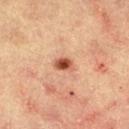Assessment: Imaged during a routine full-body skin examination; the lesion was not biopsied and no histopathology is available. Background: The patient is a male aged around 65. The lesion is on the right thigh. The lesion's longest dimension is about 2.5 mm. Captured under cross-polarized illumination. A 15 mm close-up extracted from a 3D total-body photography capture. The lesion-visualizer software estimated a mean CIELAB color near L≈48 a*≈25 b*≈32, a lesion–skin lightness drop of about 15, and a normalized lesion–skin contrast near 10.5.